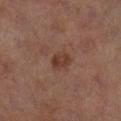follow-up = imaged on a skin check; not biopsied
image source = ~15 mm tile from a whole-body skin photo
patient = female, aged 58–62
lighting = cross-polarized
anatomic site = the right lower leg
diameter = ~2.5 mm (longest diameter)